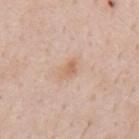biopsy status: no biopsy performed (imaged during a skin exam) | anatomic site: the mid back | subject: male, aged 58 to 62 | automated lesion analysis: an area of roughly 3 mm²; a mean CIELAB color near L≈64 a*≈18 b*≈32 and a lesion-to-skin contrast of about 6 (normalized; higher = more distinct); a nevus-likeness score of about 30/100 and a detector confidence of about 100 out of 100 that the crop contains a lesion | image: 15 mm crop, total-body photography.